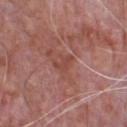Imaged during a routine full-body skin examination; the lesion was not biopsied and no histopathology is available.
Cropped from a whole-body photographic skin survey; the tile spans about 15 mm.
Located on the chest.
An algorithmic analysis of the crop reported a lesion area of about 3.5 mm². The analysis additionally found a mean CIELAB color near L≈46 a*≈26 b*≈26, a lesion–skin lightness drop of about 6, and a normalized lesion–skin contrast near 5.5. And it measured a border-irregularity index near 5/10, internal color variation of about 1 on a 0–10 scale, and a peripheral color-asymmetry measure near 0.5.
The tile uses white-light illumination.
A male patient, in their 70s.
About 2.5 mm across.